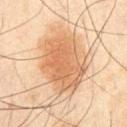Recorded during total-body skin imaging; not selected for excision or biopsy.
A male subject in their mid- to late 40s.
Located on the chest.
A 15 mm crop from a total-body photograph taken for skin-cancer surveillance.
Automated image analysis of the tile measured a lesion area of about 38 mm² and a symmetry-axis asymmetry near 0.2. The software also gave a mean CIELAB color near L≈54 a*≈17 b*≈32 and a lesion-to-skin contrast of about 7.5 (normalized; higher = more distinct). The software also gave a border-irregularity rating of about 3.5/10 and internal color variation of about 4.5 on a 0–10 scale. The analysis additionally found a classifier nevus-likeness of about 100/100.
Measured at roughly 9.5 mm in maximum diameter.